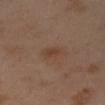Part of a total-body skin-imaging series; this lesion was reviewed on a skin check and was not flagged for biopsy. Imaged with cross-polarized lighting. The subject is a female about 40 years old. From the left arm. An algorithmic analysis of the crop reported a footprint of about 4 mm², a shape eccentricity near 0.75, and a shape-asymmetry score of about 0.15 (0 = symmetric). The software also gave a within-lesion color-variation index near 2/10. The software also gave an automated nevus-likeness rating near 30 out of 100 and lesion-presence confidence of about 100/100. This image is a 15 mm lesion crop taken from a total-body photograph. Longest diameter approximately 3 mm.